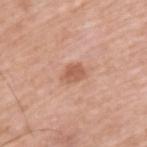<tbp_lesion>
  <biopsy_status>not biopsied; imaged during a skin examination</biopsy_status>
  <automated_metrics>
    <area_mm2_approx>4.5</area_mm2_approx>
    <eccentricity>0.7</eccentricity>
    <shape_asymmetry>0.3</shape_asymmetry>
  </automated_metrics>
  <lesion_size>
    <long_diameter_mm_approx>2.5</long_diameter_mm_approx>
  </lesion_size>
  <image>
    <source>total-body photography crop</source>
    <field_of_view_mm>15</field_of_view_mm>
  </image>
  <site>upper back</site>
  <patient>
    <sex>male</sex>
    <age_approx>65</age_approx>
  </patient>
  <lighting>white-light</lighting>
</tbp_lesion>